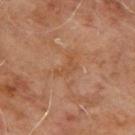follow-up: catalogued during a skin exam; not biopsied | lighting: cross-polarized illumination | location: the upper back | automated metrics: a mean CIELAB color near L≈48 a*≈22 b*≈34 and a lesion-to-skin contrast of about 5 (normalized; higher = more distinct); border irregularity of about 7.5 on a 0–10 scale and radial color variation of about 0 | subject: male, roughly 65 years of age | acquisition: total-body-photography crop, ~15 mm field of view.